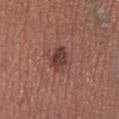No biopsy was performed on this lesion — it was imaged during a full skin examination and was not determined to be concerning. Imaged with white-light lighting. Automated tile analysis of the lesion measured an average lesion color of about L≈39 a*≈21 b*≈22 (CIELAB) and a normalized border contrast of about 8.5. The analysis additionally found a border-irregularity rating of about 2.5/10, a color-variation rating of about 3.5/10, and radial color variation of about 1.5. The software also gave a classifier nevus-likeness of about 60/100 and lesion-presence confidence of about 100/100. This image is a 15 mm lesion crop taken from a total-body photograph. The lesion is located on the left lower leg. The recorded lesion diameter is about 3 mm. The patient is a female in their 60s.